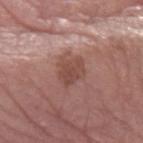Case summary:
• workup: total-body-photography surveillance lesion; no biopsy
• tile lighting: white-light
• patient: male, aged approximately 60
• acquisition: total-body-photography crop, ~15 mm field of view
• lesion size: ≈3.5 mm
• body site: the left forearm
• automated lesion analysis: internal color variation of about 2.5 on a 0–10 scale and peripheral color asymmetry of about 1